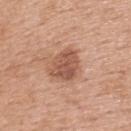Q: Was this lesion biopsied?
A: imaged on a skin check; not biopsied
Q: What are the patient's age and sex?
A: female, roughly 65 years of age
Q: Lesion location?
A: the upper back
Q: How was this image acquired?
A: ~15 mm tile from a whole-body skin photo